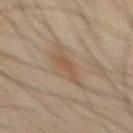{
  "biopsy_status": "not biopsied; imaged during a skin examination",
  "patient": {
    "sex": "male",
    "age_approx": 45
  },
  "lesion_size": {
    "long_diameter_mm_approx": 3.5
  },
  "site": "front of the torso",
  "automated_metrics": {
    "area_mm2_approx": 5.5,
    "cielab_L": 41,
    "cielab_a": 12,
    "cielab_b": 25,
    "vs_skin_darker_L": 6.0,
    "vs_skin_contrast_norm": 5.5,
    "color_variation_0_10": 1.0,
    "peripheral_color_asymmetry": 0.5,
    "nevus_likeness_0_100": 10,
    "lesion_detection_confidence_0_100": 100
  },
  "image": {
    "source": "total-body photography crop",
    "field_of_view_mm": 15
  },
  "lighting": "cross-polarized"
}